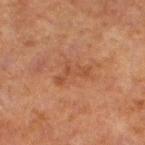This lesion was catalogued during total-body skin photography and was not selected for biopsy. A male subject in their 70s. Located on the left lower leg. Cropped from a whole-body photographic skin survey; the tile spans about 15 mm.